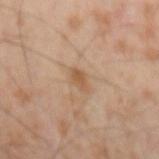biopsy status: catalogued during a skin exam; not biopsied
anatomic site: the left forearm
diameter: about 2.5 mm
acquisition: total-body-photography crop, ~15 mm field of view
illumination: cross-polarized illumination
subject: male, about 55 years old